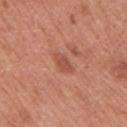Case summary:
– workup · catalogued during a skin exam; not biopsied
– patient · male, about 70 years old
– image source · 15 mm crop, total-body photography
– tile lighting · white-light illumination
– body site · the arm